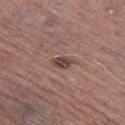Captured during whole-body skin photography for melanoma surveillance; the lesion was not biopsied.